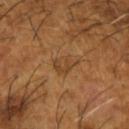Assessment: Part of a total-body skin-imaging series; this lesion was reviewed on a skin check and was not flagged for biopsy. Context: The total-body-photography lesion software estimated a footprint of about 5 mm², an outline eccentricity of about 0.6 (0 = round, 1 = elongated), and two-axis asymmetry of about 0.35. It also reported an average lesion color of about L≈43 a*≈19 b*≈36 (CIELAB), roughly 6 lightness units darker than nearby skin, and a normalized lesion–skin contrast near 5.5. The software also gave a classifier nevus-likeness of about 0/100. A male subject in their mid-60s. A 15 mm crop from a total-body photograph taken for skin-cancer surveillance. The lesion is on the left forearm. Captured under cross-polarized illumination.